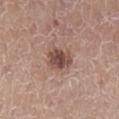Clinical impression: Part of a total-body skin-imaging series; this lesion was reviewed on a skin check and was not flagged for biopsy. Acquisition and patient details: On the right lower leg. The recorded lesion diameter is about 3.5 mm. The total-body-photography lesion software estimated an area of roughly 7.5 mm², an eccentricity of roughly 0.6, and two-axis asymmetry of about 0.2. The analysis additionally found a lesion color around L≈48 a*≈19 b*≈23 in CIELAB, about 13 CIELAB-L* units darker than the surrounding skin, and a normalized lesion–skin contrast near 9. It also reported a color-variation rating of about 3.5/10 and peripheral color asymmetry of about 1. It also reported a nevus-likeness score of about 80/100 and a lesion-detection confidence of about 100/100. A female patient in their mid- to late 40s. Imaged with white-light lighting. A 15 mm crop from a total-body photograph taken for skin-cancer surveillance.